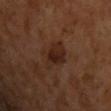Assessment: Imaged during a routine full-body skin examination; the lesion was not biopsied and no histopathology is available. Background: A male subject roughly 65 years of age. A lesion tile, about 15 mm wide, cut from a 3D total-body photograph. The lesion is located on the chest.